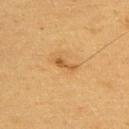{"biopsy_status": "not biopsied; imaged during a skin examination", "automated_metrics": {"cielab_L": 50, "cielab_a": 20, "cielab_b": 40, "vs_skin_darker_L": 9.0}, "patient": {"sex": "male", "age_approx": 60}, "image": {"source": "total-body photography crop", "field_of_view_mm": 15}, "lesion_size": {"long_diameter_mm_approx": 3.0}, "site": "back"}